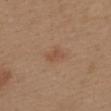Notes:
– workup — imaged on a skin check; not biopsied
– lesion diameter — ≈2.5 mm
– acquisition — ~15 mm tile from a whole-body skin photo
– subject — female, aged around 40
– illumination — white-light illumination
– location — the upper back